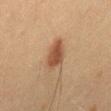automated lesion analysis: an area of roughly 6.5 mm² and a shape-asymmetry score of about 0.3 (0 = symmetric); an average lesion color of about L≈40 a*≈19 b*≈28 (CIELAB), roughly 11 lightness units darker than nearby skin, and a normalized lesion–skin contrast near 9; a border-irregularity index near 2.5/10 and peripheral color asymmetry of about 1
site: the left thigh
imaging modality: ~15 mm tile from a whole-body skin photo
lighting: cross-polarized illumination
lesion size: ~3.5 mm (longest diameter)
patient: female, roughly 40 years of age